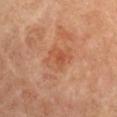{
  "biopsy_status": "not biopsied; imaged during a skin examination",
  "site": "arm",
  "lesion_size": {
    "long_diameter_mm_approx": 4.0
  },
  "automated_metrics": {
    "area_mm2_approx": 8.5,
    "eccentricity": 0.6,
    "border_irregularity_0_10": 2.5,
    "color_variation_0_10": 4.5,
    "peripheral_color_asymmetry": 1.5,
    "nevus_likeness_0_100": 0
  },
  "patient": {
    "sex": "male",
    "age_approx": 60
  },
  "lighting": "cross-polarized",
  "image": {
    "source": "total-body photography crop",
    "field_of_view_mm": 15
  }
}